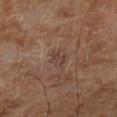• follow-up: total-body-photography surveillance lesion; no biopsy
• automated lesion analysis: a footprint of about 4 mm² and two-axis asymmetry of about 0.25; an average lesion color of about L≈39 a*≈17 b*≈23 (CIELAB), about 6 CIELAB-L* units darker than the surrounding skin, and a lesion-to-skin contrast of about 5 (normalized; higher = more distinct); border irregularity of about 2.5 on a 0–10 scale and internal color variation of about 2 on a 0–10 scale
• subject: male, aged around 65
• lesion size: ~2.5 mm (longest diameter)
• image: ~15 mm crop, total-body skin-cancer survey
• illumination: cross-polarized illumination